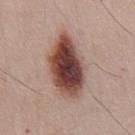Q: What is the anatomic site?
A: the front of the torso
Q: What is the lesion's diameter?
A: about 7.5 mm
Q: What lighting was used for the tile?
A: white-light illumination
Q: What kind of image is this?
A: ~15 mm crop, total-body skin-cancer survey
Q: Patient demographics?
A: male, aged 53 to 57